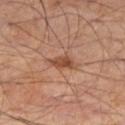Clinical impression: Recorded during total-body skin imaging; not selected for excision or biopsy. Context: On the right thigh. A male subject, about 55 years old. A roughly 15 mm field-of-view crop from a total-body skin photograph. About 3 mm across. This is a cross-polarized tile.